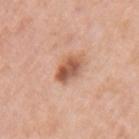The lesion was photographed on a routine skin check and not biopsied; there is no pathology result. The lesion-visualizer software estimated an automated nevus-likeness rating near 75 out of 100 and lesion-presence confidence of about 100/100. A close-up tile cropped from a whole-body skin photograph, about 15 mm across. From the left upper arm. Measured at roughly 4 mm in maximum diameter. A female patient, aged 38–42.